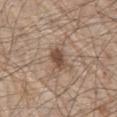On the chest. A male subject in their 80s. This image is a 15 mm lesion crop taken from a total-body photograph.